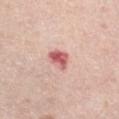notes — imaged on a skin check; not biopsied
subject — female, in their mid-60s
lesion diameter — ≈2.5 mm
site — the leg
image — ~15 mm crop, total-body skin-cancer survey